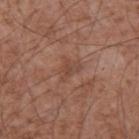The lesion was photographed on a routine skin check and not biopsied; there is no pathology result.
Located on the left upper arm.
This image is a 15 mm lesion crop taken from a total-body photograph.
Longest diameter approximately 3 mm.
This is a white-light tile.
A male subject aged 73 to 77.